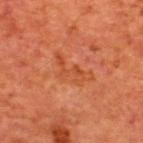The lesion was photographed on a routine skin check and not biopsied; there is no pathology result.
Longest diameter approximately 5 mm.
A 15 mm close-up extracted from a 3D total-body photography capture.
A male subject approximately 65 years of age.
The total-body-photography lesion software estimated a lesion color around L≈45 a*≈30 b*≈37 in CIELAB, about 6 CIELAB-L* units darker than the surrounding skin, and a lesion-to-skin contrast of about 5 (normalized; higher = more distinct). The analysis additionally found border irregularity of about 9.5 on a 0–10 scale, a within-lesion color-variation index near 1/10, and a peripheral color-asymmetry measure near 0.5.
The lesion is on the upper back.